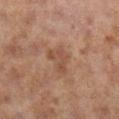Q: Was this lesion biopsied?
A: imaged on a skin check; not biopsied
Q: Patient demographics?
A: female, in their 60s
Q: Lesion location?
A: the right lower leg
Q: What is the imaging modality?
A: 15 mm crop, total-body photography